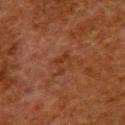Notes:
– biopsy status — total-body-photography surveillance lesion; no biopsy
– patient — female, aged approximately 50
– image — total-body-photography crop, ~15 mm field of view
– body site — the upper back